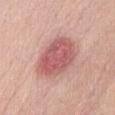The lesion is located on the front of the torso.
Imaged with white-light lighting.
The patient is a female aged approximately 50.
A 15 mm close-up tile from a total-body photography series done for melanoma screening.
An algorithmic analysis of the crop reported a footprint of about 20 mm² and a symmetry-axis asymmetry near 0.15. And it measured a lesion color around L≈58 a*≈27 b*≈23 in CIELAB and roughly 13 lightness units darker than nearby skin.
Approximately 6.5 mm at its widest.